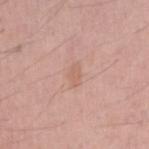Q: Is there a histopathology result?
A: catalogued during a skin exam; not biopsied
Q: Automated lesion metrics?
A: a lesion color around L≈62 a*≈21 b*≈28 in CIELAB, roughly 6 lightness units darker than nearby skin, and a lesion-to-skin contrast of about 4.5 (normalized; higher = more distinct); an automated nevus-likeness rating near 0 out of 100 and a lesion-detection confidence of about 100/100
Q: Where on the body is the lesion?
A: the right upper arm
Q: What is the lesion's diameter?
A: ≈3 mm
Q: Illumination type?
A: white-light
Q: What is the imaging modality?
A: ~15 mm crop, total-body skin-cancer survey
Q: What are the patient's age and sex?
A: male, aged around 50